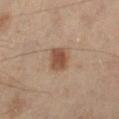Context: The lesion's longest dimension is about 3 mm. The subject is a male roughly 55 years of age. The total-body-photography lesion software estimated an average lesion color of about L≈39 a*≈15 b*≈25 (CIELAB), a lesion–skin lightness drop of about 9, and a normalized lesion–skin contrast near 8. And it measured a border-irregularity rating of about 1.5/10, a color-variation rating of about 2/10, and peripheral color asymmetry of about 0.5. The analysis additionally found lesion-presence confidence of about 100/100. A roughly 15 mm field-of-view crop from a total-body skin photograph. The lesion is located on the left lower leg. Captured under cross-polarized illumination.